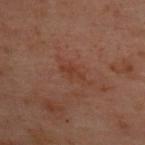biopsy status — imaged on a skin check; not biopsied
location — the upper back
image-analysis metrics — a footprint of about 3.5 mm², an outline eccentricity of about 0.8 (0 = round, 1 = elongated), and a shape-asymmetry score of about 0.25 (0 = symmetric); a border-irregularity rating of about 2.5/10, a color-variation rating of about 1.5/10, and peripheral color asymmetry of about 0.5
imaging modality — 15 mm crop, total-body photography
patient — female, aged around 55
illumination — cross-polarized illumination
lesion diameter — ~2.5 mm (longest diameter)